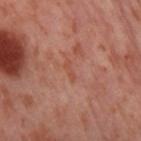Background:
A female subject, aged 53–57. A 15 mm close-up tile from a total-body photography series done for melanoma screening. Measured at roughly 2.5 mm in maximum diameter. On the right thigh.